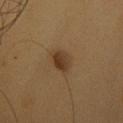Notes:
- follow-up: no biopsy performed (imaged during a skin exam)
- subject: female, roughly 35 years of age
- anatomic site: the head or neck
- acquisition: 15 mm crop, total-body photography
- lesion diameter: ~2.5 mm (longest diameter)